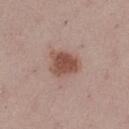Assessment: The lesion was tiled from a total-body skin photograph and was not biopsied. Acquisition and patient details: The lesion is on the left thigh. Approximately 4 mm at its widest. Imaged with white-light lighting. A female patient roughly 25 years of age. A 15 mm close-up extracted from a 3D total-body photography capture.